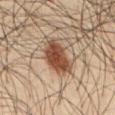Clinical summary: A region of skin cropped from a whole-body photographic capture, roughly 15 mm wide. Measured at roughly 5 mm in maximum diameter. Captured under cross-polarized illumination. On the abdomen. A male subject, in their mid- to late 30s.